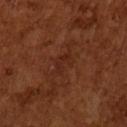This lesion was catalogued during total-body skin photography and was not selected for biopsy.
Approximately 3 mm at its widest.
A male patient in their mid-60s.
A 15 mm crop from a total-body photograph taken for skin-cancer surveillance.